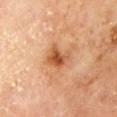Captured during whole-body skin photography for melanoma surveillance; the lesion was not biopsied.
The subject is a male about 70 years old.
The lesion is on the mid back.
Cropped from a total-body skin-imaging series; the visible field is about 15 mm.